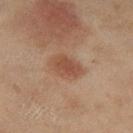Q: Was a biopsy performed?
A: no biopsy performed (imaged during a skin exam)
Q: What kind of image is this?
A: total-body-photography crop, ~15 mm field of view
Q: Who is the patient?
A: female, aged approximately 60
Q: Lesion location?
A: the left thigh